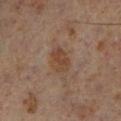Clinical summary:
The lesion is on the left lower leg. A 15 mm close-up tile from a total-body photography series done for melanoma screening. The patient is a male aged 58–62.